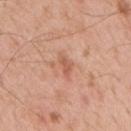Assessment: The lesion was tiled from a total-body skin photograph and was not biopsied. Background: A close-up tile cropped from a whole-body skin photograph, about 15 mm across. The total-body-photography lesion software estimated a lesion color around L≈59 a*≈25 b*≈32 in CIELAB and a normalized lesion–skin contrast near 6. The analysis additionally found a border-irregularity rating of about 2.5/10, internal color variation of about 1.5 on a 0–10 scale, and peripheral color asymmetry of about 0.5. The patient is a male roughly 55 years of age. The lesion is on the mid back. The tile uses white-light illumination. Longest diameter approximately 3 mm.